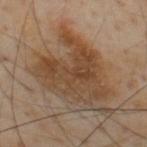The lesion was photographed on a routine skin check and not biopsied; there is no pathology result. Measured at roughly 8 mm in maximum diameter. Captured under cross-polarized illumination. On the back. The subject is a male roughly 55 years of age. A 15 mm close-up extracted from a 3D total-body photography capture.